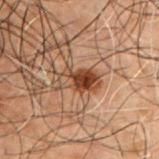Part of a total-body skin-imaging series; this lesion was reviewed on a skin check and was not flagged for biopsy.
Located on the front of the torso.
Imaged with cross-polarized lighting.
This image is a 15 mm lesion crop taken from a total-body photograph.
The subject is a male in their 50s.
Longest diameter approximately 4.5 mm.
Automated image analysis of the tile measured a lesion area of about 8.5 mm², a shape eccentricity near 0.85, and a symmetry-axis asymmetry near 0.2. And it measured a lesion color around L≈34 a*≈18 b*≈26 in CIELAB and about 10 CIELAB-L* units darker than the surrounding skin. The analysis additionally found a classifier nevus-likeness of about 100/100.